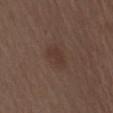<lesion>
  <biopsy_status>not biopsied; imaged during a skin examination</biopsy_status>
  <site>mid back</site>
  <lesion_size>
    <long_diameter_mm_approx>3.5</long_diameter_mm_approx>
  </lesion_size>
  <image>
    <source>total-body photography crop</source>
    <field_of_view_mm>15</field_of_view_mm>
  </image>
  <patient>
    <sex>male</sex>
    <age_approx>70</age_approx>
  </patient>
  <lighting>white-light</lighting>
</lesion>